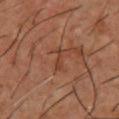Part of a total-body skin-imaging series; this lesion was reviewed on a skin check and was not flagged for biopsy. The lesion is located on the chest. A male patient approximately 55 years of age. A close-up tile cropped from a whole-body skin photograph, about 15 mm across.